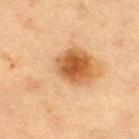Captured during whole-body skin photography for melanoma surveillance; the lesion was not biopsied. Longest diameter approximately 9.5 mm. The tile uses cross-polarized illumination. From the right thigh. The subject is a female aged approximately 40. A region of skin cropped from a whole-body photographic capture, roughly 15 mm wide.